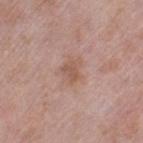Imaged during a routine full-body skin examination; the lesion was not biopsied and no histopathology is available. A female patient, roughly 40 years of age. Cropped from a whole-body photographic skin survey; the tile spans about 15 mm. From the left thigh.